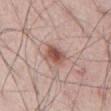biopsy status: catalogued during a skin exam; not biopsied
lighting: white-light
TBP lesion metrics: an outline eccentricity of about 0.55 (0 = round, 1 = elongated) and two-axis asymmetry of about 0.2; an average lesion color of about L≈53 a*≈22 b*≈25 (CIELAB), about 13 CIELAB-L* units darker than the surrounding skin, and a lesion-to-skin contrast of about 9 (normalized; higher = more distinct); a border-irregularity index near 2/10 and a peripheral color-asymmetry measure near 1.5; a classifier nevus-likeness of about 95/100 and lesion-presence confidence of about 100/100
imaging modality: 15 mm crop, total-body photography
site: the abdomen
size: ~2.5 mm (longest diameter)
subject: male, aged around 65